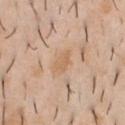Findings:
- notes — catalogued during a skin exam; not biopsied
- automated metrics — a footprint of about 4.5 mm², an eccentricity of roughly 0.8, and a shape-asymmetry score of about 0.2 (0 = symmetric); a mean CIELAB color near L≈63 a*≈18 b*≈35, roughly 7 lightness units darker than nearby skin, and a lesion-to-skin contrast of about 5.5 (normalized; higher = more distinct); internal color variation of about 1.5 on a 0–10 scale; a nevus-likeness score of about 0/100 and lesion-presence confidence of about 100/100
- lesion size — about 3 mm
- patient — male, about 40 years old
- body site — the chest
- image source — 15 mm crop, total-body photography
- illumination — white-light